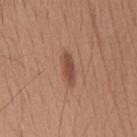Captured during whole-body skin photography for melanoma surveillance; the lesion was not biopsied.